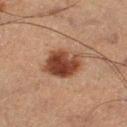Captured during whole-body skin photography for melanoma surveillance; the lesion was not biopsied. Captured under cross-polarized illumination. A 15 mm crop from a total-body photograph taken for skin-cancer surveillance. From the left thigh. A male patient in their mid- to late 70s.